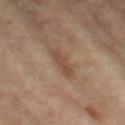Clinical impression: Imaged during a routine full-body skin examination; the lesion was not biopsied and no histopathology is available. Acquisition and patient details: Located on the left thigh. A 15 mm close-up tile from a total-body photography series done for melanoma screening. The patient is a female aged around 75. Approximately 4 mm at its widest.